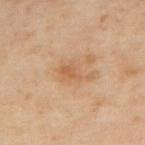Q: Is there a histopathology result?
A: no biopsy performed (imaged during a skin exam)
Q: Lesion location?
A: the upper back
Q: What is the lesion's diameter?
A: ~4 mm (longest diameter)
Q: Illumination type?
A: cross-polarized illumination
Q: Automated lesion metrics?
A: an area of roughly 6 mm², an outline eccentricity of about 0.85 (0 = round, 1 = elongated), and a shape-asymmetry score of about 0.5 (0 = symmetric); a color-variation rating of about 2/10 and radial color variation of about 0.5; an automated nevus-likeness rating near 5 out of 100 and lesion-presence confidence of about 100/100
Q: Who is the patient?
A: male, in their 50s
Q: What is the imaging modality?
A: total-body-photography crop, ~15 mm field of view